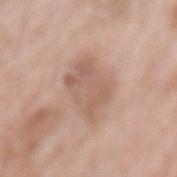Notes:
– workup — total-body-photography surveillance lesion; no biopsy
– anatomic site — the mid back
– patient — female, aged around 75
– imaging modality — 15 mm crop, total-body photography